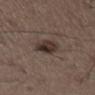No biopsy was performed on this lesion — it was imaged during a full skin examination and was not determined to be concerning. On the right thigh. A male subject, in their 50s. Captured under white-light illumination. This image is a 15 mm lesion crop taken from a total-body photograph. The lesion's longest dimension is about 3 mm.